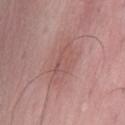Imaged during a routine full-body skin examination; the lesion was not biopsied and no histopathology is available.
A roughly 15 mm field-of-view crop from a total-body skin photograph.
A male subject, roughly 50 years of age.
Automated image analysis of the tile measured an area of roughly 12 mm², an eccentricity of roughly 0.9, and two-axis asymmetry of about 0.3.
Captured under white-light illumination.
About 6 mm across.
Located on the lower back.